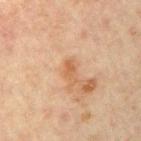| field | value |
|---|---|
| biopsy status | imaged on a skin check; not biopsied |
| subject | male, aged 43–47 |
| body site | the right upper arm |
| lesion size | ~3 mm (longest diameter) |
| lighting | cross-polarized illumination |
| image | 15 mm crop, total-body photography |